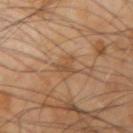Imaged during a routine full-body skin examination; the lesion was not biopsied and no histopathology is available. About 3 mm across. A 15 mm close-up extracted from a 3D total-body photography capture. A male patient, aged approximately 65. The lesion is on the right upper arm. Captured under cross-polarized illumination.